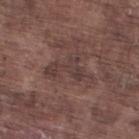workup = total-body-photography surveillance lesion; no biopsy
image = ~15 mm tile from a whole-body skin photo
body site = the right lower leg
lesion size = about 5.5 mm
subject = male, in their mid-70s
lighting = white-light illumination